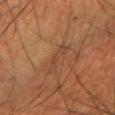Imaged during a routine full-body skin examination; the lesion was not biopsied and no histopathology is available.
A male subject, aged approximately 50.
Cropped from a whole-body photographic skin survey; the tile spans about 15 mm.
The lesion is on the right forearm.